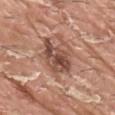Recorded during total-body skin imaging; not selected for excision or biopsy. The total-body-photography lesion software estimated a border-irregularity index near 4/10 and radial color variation of about 3. Measured at roughly 6 mm in maximum diameter. Cropped from a whole-body photographic skin survey; the tile spans about 15 mm. From the right upper arm. A male subject, about 40 years old.